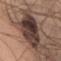<tbp_lesion>
  <biopsy_status>not biopsied; imaged during a skin examination</biopsy_status>
  <image>
    <source>total-body photography crop</source>
    <field_of_view_mm>15</field_of_view_mm>
  </image>
  <site>upper back</site>
  <patient>
    <sex>male</sex>
    <age_approx>35</age_approx>
  </patient>
</tbp_lesion>